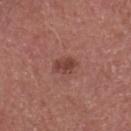Findings:
• biopsy status — total-body-photography surveillance lesion; no biopsy
• subject — male, in their mid- to late 40s
• lesion size — ≈3.5 mm
• anatomic site — the head or neck
• image — 15 mm crop, total-body photography
• lighting — white-light